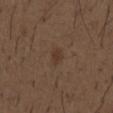Imaged with white-light lighting.
From the chest.
A 15 mm close-up extracted from a 3D total-body photography capture.
A male subject, aged approximately 50.
Measured at roughly 2.5 mm in maximum diameter.